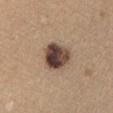Q: Was a biopsy performed?
A: no biopsy performed (imaged during a skin exam)
Q: Illumination type?
A: white-light
Q: What is the imaging modality?
A: ~15 mm crop, total-body skin-cancer survey
Q: Patient demographics?
A: female, approximately 60 years of age
Q: Lesion location?
A: the arm